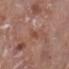Clinical impression: Imaged during a routine full-body skin examination; the lesion was not biopsied and no histopathology is available. Context: A 15 mm close-up extracted from a 3D total-body photography capture. The lesion is on the left lower leg. Longest diameter approximately 3 mm. A female subject, roughly 75 years of age. The tile uses white-light illumination.